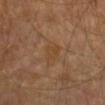workup: total-body-photography surveillance lesion; no biopsy | patient: in their mid- to late 50s | image-analysis metrics: a border-irregularity rating of about 3/10, a color-variation rating of about 2.5/10, and peripheral color asymmetry of about 1 | tile lighting: cross-polarized illumination | image source: ~15 mm crop, total-body skin-cancer survey | lesion size: about 3 mm | anatomic site: the right forearm.